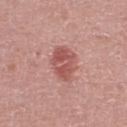location = the right lower leg; subject = male, about 75 years old; lesion diameter = ≈4 mm; lighting = white-light; image source = 15 mm crop, total-body photography.